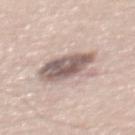A region of skin cropped from a whole-body photographic capture, roughly 15 mm wide.
The subject is a male aged 58 to 62.
On the upper back.
An algorithmic analysis of the crop reported a footprint of about 17 mm², an outline eccentricity of about 0.7 (0 = round, 1 = elongated), and a symmetry-axis asymmetry near 0.25. The software also gave an average lesion color of about L≈59 a*≈14 b*≈20 (CIELAB) and a normalized lesion–skin contrast near 9.5. The analysis additionally found border irregularity of about 3 on a 0–10 scale, internal color variation of about 6.5 on a 0–10 scale, and a peripheral color-asymmetry measure near 2.5.